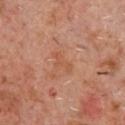No biopsy was performed on this lesion — it was imaged during a full skin examination and was not determined to be concerning. A male subject aged approximately 60. From the chest. Measured at roughly 3 mm in maximum diameter. This is a cross-polarized tile. Automated tile analysis of the lesion measured a lesion area of about 3 mm², an eccentricity of roughly 0.9, and two-axis asymmetry of about 0.55. The analysis additionally found a lesion–skin lightness drop of about 5 and a lesion-to-skin contrast of about 5 (normalized; higher = more distinct). And it measured a classifier nevus-likeness of about 0/100 and a lesion-detection confidence of about 100/100. A 15 mm crop from a total-body photograph taken for skin-cancer surveillance.